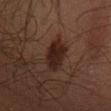Captured during whole-body skin photography for melanoma surveillance; the lesion was not biopsied.